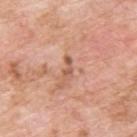workup — catalogued during a skin exam; not biopsied | patient — male, approximately 60 years of age | image source — ~15 mm crop, total-body skin-cancer survey | lighting — white-light illumination | anatomic site — the back | automated lesion analysis — an area of roughly 2 mm², a shape eccentricity near 0.95, and a shape-asymmetry score of about 0.45 (0 = symmetric) | lesion size — about 2.5 mm.